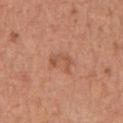This lesion was catalogued during total-body skin photography and was not selected for biopsy.
A 15 mm close-up extracted from a 3D total-body photography capture.
From the chest.
A male subject, about 65 years old.